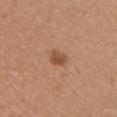Assessment: This lesion was catalogued during total-body skin photography and was not selected for biopsy. Image and clinical context: From the left upper arm. A female patient aged 43–47. A close-up tile cropped from a whole-body skin photograph, about 15 mm across. Automated tile analysis of the lesion measured a mean CIELAB color near L≈50 a*≈22 b*≈33, a lesion–skin lightness drop of about 10, and a lesion-to-skin contrast of about 7.5 (normalized; higher = more distinct). The software also gave a color-variation rating of about 3/10 and radial color variation of about 1. The software also gave an automated nevus-likeness rating near 85 out of 100 and a lesion-detection confidence of about 100/100. Imaged with white-light lighting.